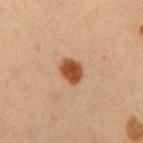The lesion was photographed on a routine skin check and not biopsied; there is no pathology result.
A close-up tile cropped from a whole-body skin photograph, about 15 mm across.
Measured at roughly 3 mm in maximum diameter.
Imaged with cross-polarized lighting.
From the right upper arm.
A male subject, approximately 60 years of age.